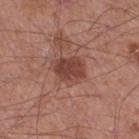No biopsy was performed on this lesion — it was imaged during a full skin examination and was not determined to be concerning.
Automated image analysis of the tile measured a lesion area of about 8.5 mm², a shape eccentricity near 0.65, and a shape-asymmetry score of about 0.2 (0 = symmetric). It also reported a border-irregularity rating of about 2/10, a within-lesion color-variation index near 3/10, and peripheral color asymmetry of about 1. The software also gave an automated nevus-likeness rating near 85 out of 100 and lesion-presence confidence of about 100/100.
The subject is a male aged 53–57.
On the right thigh.
Longest diameter approximately 3.5 mm.
Cropped from a whole-body photographic skin survey; the tile spans about 15 mm.